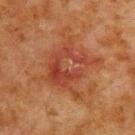– automated metrics — two-axis asymmetry of about 0.4; a mean CIELAB color near L≈34 a*≈24 b*≈28 and about 7 CIELAB-L* units darker than the surrounding skin; a border-irregularity rating of about 6.5/10, a within-lesion color-variation index near 7.5/10, and peripheral color asymmetry of about 2.5; a classifier nevus-likeness of about 0/100 and a detector confidence of about 100 out of 100 that the crop contains a lesion
– tile lighting — cross-polarized illumination
– image source — 15 mm crop, total-body photography
– site — the upper back
– diameter — ~5.5 mm (longest diameter)
– patient — male, aged 78–82